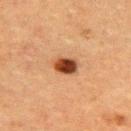<case>
<lesion_size>
  <long_diameter_mm_approx>3.5</long_diameter_mm_approx>
</lesion_size>
<image>
  <source>total-body photography crop</source>
  <field_of_view_mm>15</field_of_view_mm>
</image>
<lighting>cross-polarized</lighting>
<site>upper back</site>
<patient>
  <sex>female</sex>
  <age_approx>55</age_approx>
</patient>
</case>